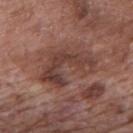Clinical impression:
The lesion was photographed on a routine skin check and not biopsied; there is no pathology result.
Context:
A 15 mm close-up tile from a total-body photography series done for melanoma screening. The lesion is located on the mid back. The tile uses white-light illumination. The patient is a male aged 68 to 72. About 7 mm across.